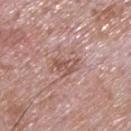This lesion was catalogued during total-body skin photography and was not selected for biopsy.
Located on the back.
The patient is a male roughly 65 years of age.
A 15 mm close-up extracted from a 3D total-body photography capture.